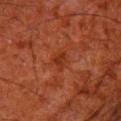Clinical impression: Recorded during total-body skin imaging; not selected for excision or biopsy. Acquisition and patient details: A male patient aged 78 to 82. A 15 mm crop from a total-body photograph taken for skin-cancer surveillance. The lesion is on the right thigh. Automated image analysis of the tile measured a border-irregularity index near 3.5/10 and radial color variation of about 0.5. The analysis additionally found an automated nevus-likeness rating near 0 out of 100 and a detector confidence of about 100 out of 100 that the crop contains a lesion. The tile uses cross-polarized illumination.